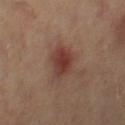biopsy status = total-body-photography surveillance lesion; no biopsy
anatomic site = the right thigh
patient = female, approximately 40 years of age
lighting = cross-polarized illumination
imaging modality = total-body-photography crop, ~15 mm field of view